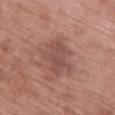Q: Is there a histopathology result?
A: total-body-photography surveillance lesion; no biopsy
Q: Who is the patient?
A: female, aged 63 to 67
Q: How large is the lesion?
A: ~4.5 mm (longest diameter)
Q: Lesion location?
A: the upper back
Q: How was this image acquired?
A: ~15 mm crop, total-body skin-cancer survey
Q: What did automated image analysis measure?
A: a footprint of about 12 mm², a shape eccentricity near 0.7, and a symmetry-axis asymmetry near 0.35; a color-variation rating of about 3/10 and peripheral color asymmetry of about 1
Q: How was the tile lit?
A: white-light illumination